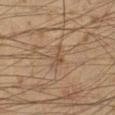Case summary:
• patient: male, aged 38–42
• image source: ~15 mm tile from a whole-body skin photo
• site: the left lower leg